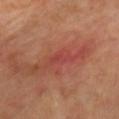The lesion was tiled from a total-body skin photograph and was not biopsied. Cropped from a total-body skin-imaging series; the visible field is about 15 mm. The subject is a male aged around 65. The lesion is on the chest. About 4 mm across. The tile uses cross-polarized illumination.